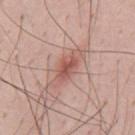<lesion>
<biopsy_status>not biopsied; imaged during a skin examination</biopsy_status>
<site>mid back</site>
<patient>
  <sex>male</sex>
  <age_approx>35</age_approx>
</patient>
<image>
  <source>total-body photography crop</source>
  <field_of_view_mm>15</field_of_view_mm>
</image>
</lesion>